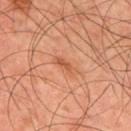{"biopsy_status": "not biopsied; imaged during a skin examination", "image": {"source": "total-body photography crop", "field_of_view_mm": 15}, "lesion_size": {"long_diameter_mm_approx": 3.0}, "automated_metrics": {"area_mm2_approx": 3.5, "eccentricity": 0.9, "shape_asymmetry": 0.25, "cielab_L": 53, "cielab_a": 26, "cielab_b": 35, "vs_skin_darker_L": 8.0, "vs_skin_contrast_norm": 6.0, "nevus_likeness_0_100": 0, "lesion_detection_confidence_0_100": 100}, "site": "upper back", "patient": {"sex": "male", "age_approx": 45}}